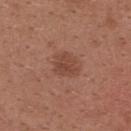Notes:
• biopsy status: no biopsy performed (imaged during a skin exam)
• automated lesion analysis: border irregularity of about 2.5 on a 0–10 scale and a peripheral color-asymmetry measure near 0.5
• site: the back
• lesion diameter: ~3 mm (longest diameter)
• imaging modality: ~15 mm crop, total-body skin-cancer survey
• lighting: white-light illumination
• patient: female, aged approximately 30